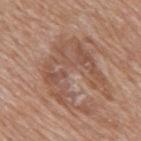Captured under white-light illumination. The total-body-photography lesion software estimated a lesion area of about 27 mm² and a symmetry-axis asymmetry near 0.5. It also reported an average lesion color of about L≈52 a*≈19 b*≈27 (CIELAB), a lesion–skin lightness drop of about 7, and a normalized lesion–skin contrast near 5.5. It also reported a border-irregularity index near 8/10, a within-lesion color-variation index near 5/10, and radial color variation of about 1.5. The software also gave a nevus-likeness score of about 0/100. The subject is a male aged approximately 60. A close-up tile cropped from a whole-body skin photograph, about 15 mm across. The lesion is located on the mid back. Measured at roughly 7 mm in maximum diameter.